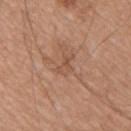Clinical impression:
No biopsy was performed on this lesion — it was imaged during a full skin examination and was not determined to be concerning.
Image and clinical context:
The lesion-visualizer software estimated a border-irregularity index near 6.5/10 and a color-variation rating of about 0.5/10. It also reported lesion-presence confidence of about 95/100. The lesion is located on the upper back. A male patient, aged around 40. Longest diameter approximately 3 mm. A lesion tile, about 15 mm wide, cut from a 3D total-body photograph.